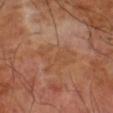{"biopsy_status": "not biopsied; imaged during a skin examination", "automated_metrics": {"area_mm2_approx": 11.0, "eccentricity": 0.8, "shape_asymmetry": 0.45, "vs_skin_contrast_norm": 4.5, "nevus_likeness_0_100": 0, "lesion_detection_confidence_0_100": 100}, "site": "right upper arm", "patient": {"sex": "male", "age_approx": 70}, "lesion_size": {"long_diameter_mm_approx": 5.5}, "image": {"source": "total-body photography crop", "field_of_view_mm": 15}}